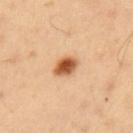Assessment:
Recorded during total-body skin imaging; not selected for excision or biopsy.
Context:
A roughly 15 mm field-of-view crop from a total-body skin photograph. About 3 mm across. Located on the left upper arm. A male subject, about 40 years old.